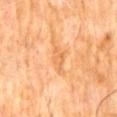{
  "biopsy_status": "not biopsied; imaged during a skin examination",
  "automated_metrics": {
    "eccentricity": 0.85,
    "shape_asymmetry": 0.25
  },
  "image": {
    "source": "total-body photography crop",
    "field_of_view_mm": 15
  },
  "lighting": "cross-polarized",
  "site": "abdomen",
  "patient": {
    "sex": "male",
    "age_approx": 60
  }
}